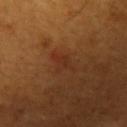Impression: This lesion was catalogued during total-body skin photography and was not selected for biopsy. Background: From the left upper arm. The lesion's longest dimension is about 3.5 mm. Captured under cross-polarized illumination. A male patient aged 63–67. A region of skin cropped from a whole-body photographic capture, roughly 15 mm wide.